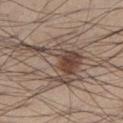Impression: Part of a total-body skin-imaging series; this lesion was reviewed on a skin check and was not flagged for biopsy. Acquisition and patient details: About 9 mm across. A close-up tile cropped from a whole-body skin photograph, about 15 mm across. A male subject in their mid- to late 50s. Automated tile analysis of the lesion measured border irregularity of about 10 on a 0–10 scale, a within-lesion color-variation index near 6/10, and a peripheral color-asymmetry measure near 2. The tile uses white-light illumination. From the left lower leg.